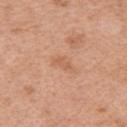Notes:
- notes — imaged on a skin check; not biopsied
- location — the arm
- acquisition — ~15 mm crop, total-body skin-cancer survey
- patient — female, aged around 50
- automated metrics — border irregularity of about 4 on a 0–10 scale, a color-variation rating of about 1.5/10, and a peripheral color-asymmetry measure near 0.5; a classifier nevus-likeness of about 0/100 and a detector confidence of about 100 out of 100 that the crop contains a lesion